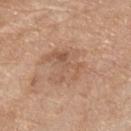{
  "biopsy_status": "not biopsied; imaged during a skin examination",
  "lighting": "white-light",
  "image": {
    "source": "total-body photography crop",
    "field_of_view_mm": 15
  },
  "automated_metrics": {
    "area_mm2_approx": 18.0,
    "shape_asymmetry": 0.2,
    "cielab_L": 57,
    "cielab_a": 19,
    "cielab_b": 31,
    "vs_skin_darker_L": 7.0,
    "vs_skin_contrast_norm": 5.0,
    "border_irregularity_0_10": 4.0,
    "peripheral_color_asymmetry": 1.5
  },
  "site": "front of the torso",
  "patient": {
    "sex": "male",
    "age_approx": 80
  },
  "lesion_size": {
    "long_diameter_mm_approx": 5.5
  }
}